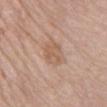workup: total-body-photography surveillance lesion; no biopsy | lesion diameter: ~4 mm (longest diameter) | anatomic site: the abdomen | lighting: white-light illumination | acquisition: ~15 mm tile from a whole-body skin photo | subject: female, aged 73–77 | TBP lesion metrics: an area of roughly 8 mm², a shape eccentricity near 0.75, and a shape-asymmetry score of about 0.15 (0 = symmetric); a lesion color around L≈59 a*≈18 b*≈30 in CIELAB, about 7 CIELAB-L* units darker than the surrounding skin, and a normalized lesion–skin contrast near 6; a border-irregularity index near 2/10 and a peripheral color-asymmetry measure near 1; a lesion-detection confidence of about 100/100.